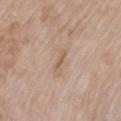Image and clinical context:
Automated image analysis of the tile measured an average lesion color of about L≈58 a*≈16 b*≈29 (CIELAB), roughly 8 lightness units darker than nearby skin, and a normalized border contrast of about 5.5. It also reported border irregularity of about 3.5 on a 0–10 scale and a color-variation rating of about 0/10. The analysis additionally found a nevus-likeness score of about 0/100. Located on the front of the torso. The subject is a female in their mid-70s. Cropped from a whole-body photographic skin survey; the tile spans about 15 mm. About 2.5 mm across.